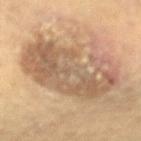Imaged during a routine full-body skin examination; the lesion was not biopsied and no histopathology is available.